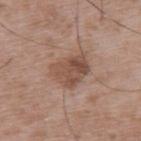biopsy status: total-body-photography surveillance lesion; no biopsy
location: the upper back
tile lighting: white-light illumination
patient: male, in their 50s
acquisition: ~15 mm tile from a whole-body skin photo